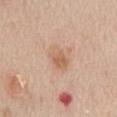Clinical impression: Imaged during a routine full-body skin examination; the lesion was not biopsied and no histopathology is available. Image and clinical context: The recorded lesion diameter is about 3.5 mm. On the abdomen. A male subject aged 58–62. This image is a 15 mm lesion crop taken from a total-body photograph. The tile uses white-light illumination. An algorithmic analysis of the crop reported about 8 CIELAB-L* units darker than the surrounding skin and a normalized lesion–skin contrast near 6. And it measured an automated nevus-likeness rating near 45 out of 100.